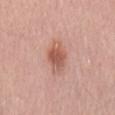notes = total-body-photography surveillance lesion; no biopsy
patient = male, aged 53–57
illumination = white-light illumination
image source = ~15 mm tile from a whole-body skin photo
size = ≈4 mm
location = the back